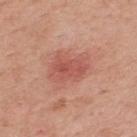Context: A male patient, aged 53 to 57. The lesion's longest dimension is about 4.5 mm. A close-up tile cropped from a whole-body skin photograph, about 15 mm across. The lesion is on the upper back.